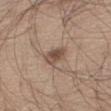Recorded during total-body skin imaging; not selected for excision or biopsy. Cropped from a whole-body photographic skin survey; the tile spans about 15 mm. The lesion's longest dimension is about 3 mm. A male subject aged approximately 60. An algorithmic analysis of the crop reported a lesion color around L≈49 a*≈16 b*≈26 in CIELAB, a lesion–skin lightness drop of about 11, and a normalized lesion–skin contrast near 8.5. And it measured a within-lesion color-variation index near 3.5/10 and a peripheral color-asymmetry measure near 1. This is a white-light tile. From the right thigh.